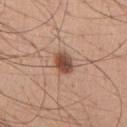Q: Is there a histopathology result?
A: no biopsy performed (imaged during a skin exam)
Q: What are the patient's age and sex?
A: male, in their mid-50s
Q: Where on the body is the lesion?
A: the chest
Q: What is the imaging modality?
A: 15 mm crop, total-body photography
Q: What is the lesion's diameter?
A: ≈3 mm
Q: What lighting was used for the tile?
A: white-light